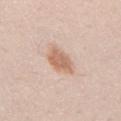| key | value |
|---|---|
| image source | 15 mm crop, total-body photography |
| location | the mid back |
| lesion diameter | ~4 mm (longest diameter) |
| patient | male, aged 33–37 |
| illumination | white-light illumination |